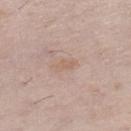{"biopsy_status": "not biopsied; imaged during a skin examination", "automated_metrics": {"area_mm2_approx": 3.0, "shape_asymmetry": 0.25, "cielab_L": 61, "cielab_a": 16, "cielab_b": 27, "vs_skin_darker_L": 6.0, "vs_skin_contrast_norm": 4.5, "nevus_likeness_0_100": 0, "lesion_detection_confidence_0_100": 100}, "lighting": "white-light", "patient": {"sex": "female", "age_approx": 60}, "site": "left lower leg", "image": {"source": "total-body photography crop", "field_of_view_mm": 15}, "lesion_size": {"long_diameter_mm_approx": 2.5}}